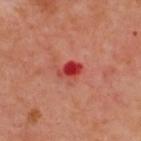{
  "lesion_size": {
    "long_diameter_mm_approx": 3.0
  },
  "image": {
    "source": "total-body photography crop",
    "field_of_view_mm": 15
  },
  "lighting": "cross-polarized",
  "patient": {
    "sex": "male",
    "age_approx": 50
  },
  "site": "upper back"
}